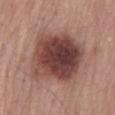No biopsy was performed on this lesion — it was imaged during a full skin examination and was not determined to be concerning. This is a white-light tile. The subject is a male roughly 65 years of age. Cropped from a total-body skin-imaging series; the visible field is about 15 mm. The total-body-photography lesion software estimated a footprint of about 34 mm² and an outline eccentricity of about 0.45 (0 = round, 1 = elongated). It also reported a mean CIELAB color near L≈42 a*≈22 b*≈22, about 16 CIELAB-L* units darker than the surrounding skin, and a normalized border contrast of about 12. The lesion is located on the back.